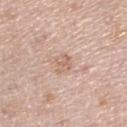biopsy status: total-body-photography surveillance lesion; no biopsy | tile lighting: white-light | body site: the right lower leg | imaging modality: total-body-photography crop, ~15 mm field of view | diameter: ≈2.5 mm | patient: female, roughly 65 years of age | image-analysis metrics: a lesion area of about 4 mm², a shape eccentricity near 0.65, and a shape-asymmetry score of about 0.25 (0 = symmetric); a lesion color around L≈63 a*≈19 b*≈28 in CIELAB, a lesion–skin lightness drop of about 8, and a normalized border contrast of about 5.5.